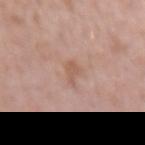Part of a total-body skin-imaging series; this lesion was reviewed on a skin check and was not flagged for biopsy. The patient is a male about 60 years old. Cropped from a total-body skin-imaging series; the visible field is about 15 mm. On the right forearm. The tile uses white-light illumination. The lesion-visualizer software estimated an area of roughly 3 mm² and a symmetry-axis asymmetry near 0.45. It also reported a lesion color around L≈57 a*≈20 b*≈28 in CIELAB and a lesion-to-skin contrast of about 5.5 (normalized; higher = more distinct). The software also gave an automated nevus-likeness rating near 0 out of 100.